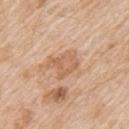Case summary:
• follow-up · total-body-photography surveillance lesion; no biopsy
• image source · ~15 mm tile from a whole-body skin photo
• diameter · ≈4 mm
• lighting · white-light illumination
• location · the arm
• subject · male, in their mid-60s